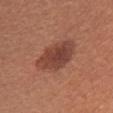Q: Was this lesion biopsied?
A: no biopsy performed (imaged during a skin exam)
Q: Lesion location?
A: the chest
Q: Illumination type?
A: white-light illumination
Q: How was this image acquired?
A: ~15 mm tile from a whole-body skin photo
Q: Automated lesion metrics?
A: a mean CIELAB color near L≈43 a*≈25 b*≈28, a lesion–skin lightness drop of about 11, and a normalized lesion–skin contrast near 8.5; a border-irregularity index near 2/10, internal color variation of about 4.5 on a 0–10 scale, and a peripheral color-asymmetry measure near 1.5
Q: Who is the patient?
A: female, aged approximately 35
Q: How large is the lesion?
A: ≈6 mm